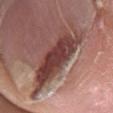The lesion was tiled from a total-body skin photograph and was not biopsied.
A male patient aged 43–47.
Imaged with white-light lighting.
Cropped from a whole-body photographic skin survey; the tile spans about 15 mm.
The lesion is on the right lower leg.
The recorded lesion diameter is about 9.5 mm.
Automated image analysis of the tile measured a mean CIELAB color near L≈41 a*≈23 b*≈22 and a lesion–skin lightness drop of about 15.